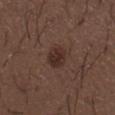Q: Was this lesion biopsied?
A: total-body-photography surveillance lesion; no biopsy
Q: What is the anatomic site?
A: the mid back
Q: Who is the patient?
A: male, aged 48–52
Q: What is the imaging modality?
A: total-body-photography crop, ~15 mm field of view